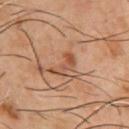Notes:
• workup · no biopsy performed (imaged during a skin exam)
• size · ≈3.5 mm
• anatomic site · the chest
• imaging modality · 15 mm crop, total-body photography
• patient · male, aged around 55
• lighting · cross-polarized illumination